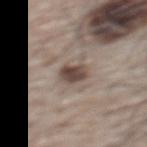notes = imaged on a skin check; not biopsied | automated lesion analysis = a mean CIELAB color near L≈45 a*≈14 b*≈21, a lesion–skin lightness drop of about 14, and a normalized border contrast of about 10.5; border irregularity of about 2.5 on a 0–10 scale and peripheral color asymmetry of about 1; a classifier nevus-likeness of about 90/100 and a lesion-detection confidence of about 100/100 | location = the upper back | patient = male, aged 63 to 67 | imaging modality = ~15 mm tile from a whole-body skin photo.